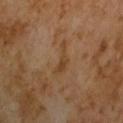workup = no biopsy performed (imaged during a skin exam); image source = total-body-photography crop, ~15 mm field of view; patient = male, approximately 65 years of age.